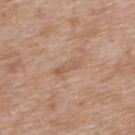follow-up: total-body-photography surveillance lesion; no biopsy | imaging modality: ~15 mm crop, total-body skin-cancer survey | body site: the upper back | patient: female, in their 40s | automated lesion analysis: roughly 7 lightness units darker than nearby skin and a lesion-to-skin contrast of about 5 (normalized; higher = more distinct); an automated nevus-likeness rating near 0 out of 100 and lesion-presence confidence of about 100/100 | lesion diameter: ≈3.5 mm.